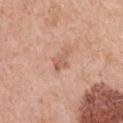Background: The lesion-visualizer software estimated border irregularity of about 4 on a 0–10 scale, internal color variation of about 2 on a 0–10 scale, and a peripheral color-asymmetry measure near 0.5. The lesion is located on the chest. The recorded lesion diameter is about 3 mm. The patient is a female in their 40s. A roughly 15 mm field-of-view crop from a total-body skin photograph.